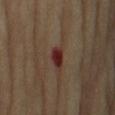<lesion>
  <patient>
    <sex>male</sex>
    <age_approx>85+</age_approx>
  </patient>
  <lesion_size>
    <long_diameter_mm_approx>2.5</long_diameter_mm_approx>
  </lesion_size>
  <image>
    <source>total-body photography crop</source>
    <field_of_view_mm>15</field_of_view_mm>
  </image>
  <automated_metrics>
    <area_mm2_approx>4.0</area_mm2_approx>
    <eccentricity>0.75</eccentricity>
    <cielab_L>27</cielab_L>
    <cielab_a>23</cielab_a>
    <cielab_b>21</cielab_b>
    <vs_skin_darker_L>11.0</vs_skin_darker_L>
    <vs_skin_contrast_norm>12.0</vs_skin_contrast_norm>
    <border_irregularity_0_10>2.5</border_irregularity_0_10>
    <peripheral_color_asymmetry>1.0</peripheral_color_asymmetry>
  </automated_metrics>
  <site>right upper arm</site>
  <lighting>cross-polarized</lighting>
</lesion>